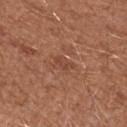acquisition = total-body-photography crop, ~15 mm field of view
site = the right upper arm
image-analysis metrics = internal color variation of about 0 on a 0–10 scale and peripheral color asymmetry of about 0; lesion-presence confidence of about 95/100
lesion diameter = ~2.5 mm (longest diameter)
subject = female, aged 38–42